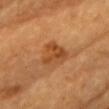Imaged during a routine full-body skin examination; the lesion was not biopsied and no histopathology is available. From the chest. Measured at roughly 4 mm in maximum diameter. A female patient roughly 65 years of age. Cropped from a whole-body photographic skin survey; the tile spans about 15 mm. Imaged with cross-polarized lighting.